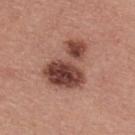biopsy status=imaged on a skin check; not biopsied
acquisition=~15 mm tile from a whole-body skin photo
subject=male, aged around 40
site=the upper back